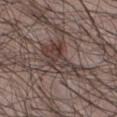<tbp_lesion>
<biopsy_status>not biopsied; imaged during a skin examination</biopsy_status>
<lighting>white-light</lighting>
<automated_metrics>
  <area_mm2_approx>13.0</area_mm2_approx>
  <eccentricity>0.85</eccentricity>
  <shape_asymmetry>0.4</shape_asymmetry>
  <vs_skin_contrast_norm>7.0</vs_skin_contrast_norm>
  <border_irregularity_0_10>5.0</border_irregularity_0_10>
  <color_variation_0_10>7.0</color_variation_0_10>
  <nevus_likeness_0_100>10</nevus_likeness_0_100>
  <lesion_detection_confidence_0_100>70</lesion_detection_confidence_0_100>
</automated_metrics>
<lesion_size>
  <long_diameter_mm_approx>5.5</long_diameter_mm_approx>
</lesion_size>
<image>
  <source>total-body photography crop</source>
  <field_of_view_mm>15</field_of_view_mm>
</image>
<site>chest</site>
<patient>
  <sex>male</sex>
  <age_approx>40</age_approx>
</patient>
</tbp_lesion>